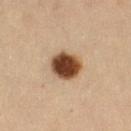The lesion was tiled from a total-body skin photograph and was not biopsied. A 15 mm close-up extracted from a 3D total-body photography capture. A female subject, aged around 40. The lesion is on the left leg. The tile uses cross-polarized illumination. Approximately 4 mm at its widest.